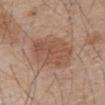Notes:
• notes: catalogued during a skin exam; not biopsied